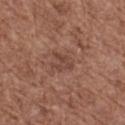A female patient aged 73–77. The lesion is on the right thigh. Measured at roughly 2.5 mm in maximum diameter. A lesion tile, about 15 mm wide, cut from a 3D total-body photograph. The tile uses white-light illumination.